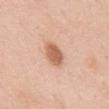Recorded during total-body skin imaging; not selected for excision or biopsy. Captured under white-light illumination. On the mid back. A male patient, aged 58 to 62. A close-up tile cropped from a whole-body skin photograph, about 15 mm across. An algorithmic analysis of the crop reported an eccentricity of roughly 0.7 and a shape-asymmetry score of about 0.15 (0 = symmetric).